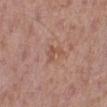Findings:
– notes: no biopsy performed (imaged during a skin exam)
– patient: male, roughly 80 years of age
– lesion diameter: ~3 mm (longest diameter)
– image source: 15 mm crop, total-body photography
– location: the left lower leg
– automated metrics: an area of roughly 3.5 mm² and a shape eccentricity near 0.75